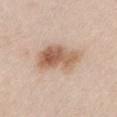follow-up: total-body-photography surveillance lesion; no biopsy | size: about 6.5 mm | automated lesion analysis: a shape eccentricity near 0.85 and a symmetry-axis asymmetry near 0.25; a classifier nevus-likeness of about 80/100 and a lesion-detection confidence of about 100/100 | body site: the chest | acquisition: total-body-photography crop, ~15 mm field of view | illumination: white-light illumination | patient: male, approximately 35 years of age.